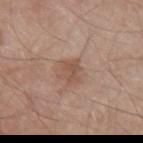biopsy status=total-body-photography surveillance lesion; no biopsy | body site=the right upper arm | imaging modality=total-body-photography crop, ~15 mm field of view | illumination=white-light illumination | subject=male, approximately 80 years of age.